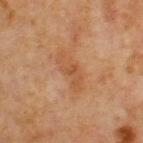Q: Was a biopsy performed?
A: total-body-photography surveillance lesion; no biopsy
Q: Illumination type?
A: cross-polarized illumination
Q: Who is the patient?
A: male, aged 63 to 67
Q: What is the lesion's diameter?
A: about 5 mm
Q: What kind of image is this?
A: ~15 mm tile from a whole-body skin photo
Q: Lesion location?
A: the chest